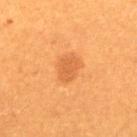• workup · total-body-photography surveillance lesion; no biopsy
• imaging modality · total-body-photography crop, ~15 mm field of view
• diameter · ≈3 mm
• automated lesion analysis · a footprint of about 5.5 mm² and a shape eccentricity near 0.7; about 8 CIELAB-L* units darker than the surrounding skin
• subject · female, aged 28 to 32
• lighting · cross-polarized
• anatomic site · the upper back